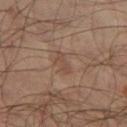Q: How was the tile lit?
A: cross-polarized illumination
Q: What are the patient's age and sex?
A: male, aged 63–67
Q: Where on the body is the lesion?
A: the left thigh
Q: Automated lesion metrics?
A: an area of roughly 2.5 mm² and a symmetry-axis asymmetry near 0.4
Q: Lesion size?
A: ≈3 mm
Q: What is the imaging modality?
A: 15 mm crop, total-body photography